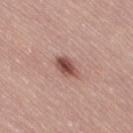biopsy status: no biopsy performed (imaged during a skin exam)
TBP lesion metrics: an outline eccentricity of about 0.8 (0 = round, 1 = elongated); a lesion color around L≈49 a*≈23 b*≈25 in CIELAB and a normalized border contrast of about 10; a border-irregularity rating of about 1.5/10 and radial color variation of about 1.5; an automated nevus-likeness rating near 95 out of 100 and a detector confidence of about 100 out of 100 that the crop contains a lesion
site: the right thigh
subject: female, aged 48–52
image: total-body-photography crop, ~15 mm field of view